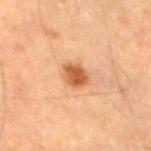Captured during whole-body skin photography for melanoma surveillance; the lesion was not biopsied.
The tile uses cross-polarized illumination.
Approximately 3.5 mm at its widest.
The lesion is located on the leg.
Cropped from a total-body skin-imaging series; the visible field is about 15 mm.
A male subject approximately 85 years of age or older.